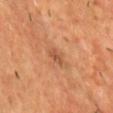Imaged during a routine full-body skin examination; the lesion was not biopsied and no histopathology is available. The recorded lesion diameter is about 2.5 mm. From the front of the torso. The subject is a male approximately 60 years of age. A close-up tile cropped from a whole-body skin photograph, about 15 mm across.